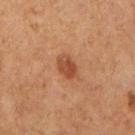biopsy status: no biopsy performed (imaged during a skin exam) | patient: female, roughly 60 years of age | body site: the right upper arm | automated lesion analysis: a lesion area of about 4.5 mm² and a symmetry-axis asymmetry near 0.15; a border-irregularity index near 1.5/10 and internal color variation of about 3 on a 0–10 scale; an automated nevus-likeness rating near 85 out of 100 and a lesion-detection confidence of about 100/100 | acquisition: total-body-photography crop, ~15 mm field of view.